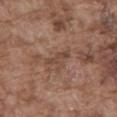Assessment:
Captured during whole-body skin photography for melanoma surveillance; the lesion was not biopsied.
Image and clinical context:
The subject is a male about 75 years old. Imaged with white-light lighting. From the abdomen. A region of skin cropped from a whole-body photographic capture, roughly 15 mm wide. The lesion-visualizer software estimated an average lesion color of about L≈45 a*≈19 b*≈27 (CIELAB) and a lesion–skin lightness drop of about 7. It also reported a classifier nevus-likeness of about 0/100 and lesion-presence confidence of about 95/100.